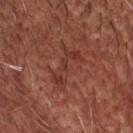Assessment:
The lesion was photographed on a routine skin check and not biopsied; there is no pathology result.
Image and clinical context:
A close-up tile cropped from a whole-body skin photograph, about 15 mm across. The tile uses white-light illumination. The lesion-visualizer software estimated a lesion area of about 7.5 mm², an eccentricity of roughly 0.9, and a symmetry-axis asymmetry near 0.4. And it measured a mean CIELAB color near L≈37 a*≈26 b*≈26, a lesion–skin lightness drop of about 6, and a lesion-to-skin contrast of about 5.5 (normalized; higher = more distinct). The lesion is located on the head or neck. A male subject, roughly 65 years of age.